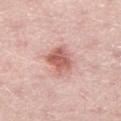Impression:
Captured during whole-body skin photography for melanoma surveillance; the lesion was not biopsied.
Clinical summary:
About 3.5 mm across. The tile uses white-light illumination. The lesion is on the right thigh. A male patient about 50 years old. This image is a 15 mm lesion crop taken from a total-body photograph.